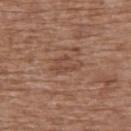<record>
<biopsy_status>not biopsied; imaged during a skin examination</biopsy_status>
<patient>
  <sex>female</sex>
  <age_approx>75</age_approx>
</patient>
<site>upper back</site>
<image>
  <source>total-body photography crop</source>
  <field_of_view_mm>15</field_of_view_mm>
</image>
</record>